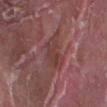Assessment:
Part of a total-body skin-imaging series; this lesion was reviewed on a skin check and was not flagged for biopsy.
Acquisition and patient details:
Measured at roughly 4 mm in maximum diameter. The lesion is on the right forearm. The tile uses white-light illumination. A 15 mm close-up extracted from a 3D total-body photography capture. The subject is a male aged 38–42. The total-body-photography lesion software estimated a footprint of about 7.5 mm², a shape eccentricity near 0.8, and two-axis asymmetry of about 0.3.